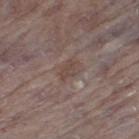Imaged during a routine full-body skin examination; the lesion was not biopsied and no histopathology is available.